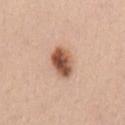| field | value |
|---|---|
| notes | no biopsy performed (imaged during a skin exam) |
| acquisition | total-body-photography crop, ~15 mm field of view |
| TBP lesion metrics | a border-irregularity rating of about 1.5/10, a within-lesion color-variation index near 8/10, and a peripheral color-asymmetry measure near 2.5 |
| lesion diameter | ~4 mm (longest diameter) |
| location | the left upper arm |
| patient | female, approximately 40 years of age |
| illumination | white-light illumination |